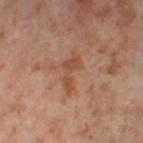No biopsy was performed on this lesion — it was imaged during a full skin examination and was not determined to be concerning.
Captured under cross-polarized illumination.
Approximately 4.5 mm at its widest.
A region of skin cropped from a whole-body photographic capture, roughly 15 mm wide.
A female patient, approximately 55 years of age.
Located on the left thigh.